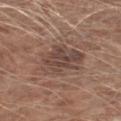notes: no biopsy performed (imaged during a skin exam) | illumination: white-light illumination | lesion size: ~6 mm (longest diameter) | image-analysis metrics: a border-irregularity rating of about 3.5/10, internal color variation of about 4.5 on a 0–10 scale, and a peripheral color-asymmetry measure near 1.5 | patient: male, in their 60s | image source: 15 mm crop, total-body photography | anatomic site: the right forearm.